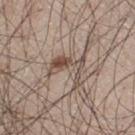This lesion was catalogued during total-body skin photography and was not selected for biopsy. The patient is a male aged approximately 30. A 15 mm close-up extracted from a 3D total-body photography capture. Automated image analysis of the tile measured a footprint of about 7.5 mm², a shape eccentricity near 0.7, and two-axis asymmetry of about 0.6. The analysis additionally found roughly 10 lightness units darker than nearby skin and a lesion-to-skin contrast of about 7.5 (normalized; higher = more distinct). On the left thigh. Longest diameter approximately 4 mm. Imaged with white-light lighting.